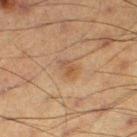Q: Was a biopsy performed?
A: no biopsy performed (imaged during a skin exam)
Q: Who is the patient?
A: male, in their 60s
Q: What is the anatomic site?
A: the right thigh
Q: What did automated image analysis measure?
A: a footprint of about 3.5 mm², an outline eccentricity of about 0.75 (0 = round, 1 = elongated), and a shape-asymmetry score of about 0.3 (0 = symmetric)
Q: How was this image acquired?
A: total-body-photography crop, ~15 mm field of view
Q: Lesion size?
A: ~2.5 mm (longest diameter)
Q: How was the tile lit?
A: cross-polarized